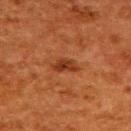Image and clinical context: A 15 mm close-up extracted from a 3D total-body photography capture. The lesion is located on the upper back. A female patient, aged 48–52.